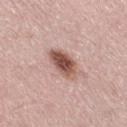lighting: white-light illumination | body site: the leg | diameter: ≈4 mm | patient: male, aged approximately 60 | TBP lesion metrics: a lesion area of about 8 mm², a shape eccentricity near 0.75, and a shape-asymmetry score of about 0.2 (0 = symmetric) | image source: total-body-photography crop, ~15 mm field of view.